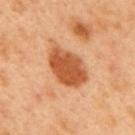Impression:
No biopsy was performed on this lesion — it was imaged during a full skin examination and was not determined to be concerning.
Context:
A male patient, aged 48 to 52. This is a cross-polarized tile. The lesion is on the back. A 15 mm crop from a total-body photograph taken for skin-cancer surveillance. About 5.5 mm across.